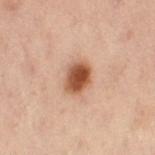A female patient roughly 55 years of age.
On the abdomen.
A 15 mm close-up tile from a total-body photography series done for melanoma screening.